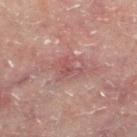Imaged during a routine full-body skin examination; the lesion was not biopsied and no histopathology is available. Measured at roughly 3.5 mm in maximum diameter. A male patient, roughly 60 years of age. A lesion tile, about 15 mm wide, cut from a 3D total-body photograph. Automated image analysis of the tile measured a lesion area of about 5 mm² and a shape eccentricity near 0.75. The tile uses cross-polarized illumination. On the left lower leg.